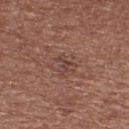The lesion was tiled from a total-body skin photograph and was not biopsied. Imaged with white-light lighting. A female subject approximately 55 years of age. From the leg. A roughly 15 mm field-of-view crop from a total-body skin photograph. Longest diameter approximately 2.5 mm.